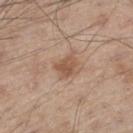This lesion was catalogued during total-body skin photography and was not selected for biopsy. The recorded lesion diameter is about 3 mm. A 15 mm close-up extracted from a 3D total-body photography capture. The total-body-photography lesion software estimated a border-irregularity index near 3/10, a color-variation rating of about 2.5/10, and peripheral color asymmetry of about 1. The analysis additionally found a classifier nevus-likeness of about 30/100 and a lesion-detection confidence of about 100/100. A male subject approximately 60 years of age. From the left thigh. Captured under white-light illumination.